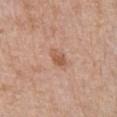workup = total-body-photography surveillance lesion; no biopsy
site = the chest
tile lighting = white-light illumination
diameter = about 2.5 mm
acquisition = ~15 mm tile from a whole-body skin photo
subject = male, about 70 years old